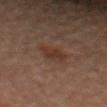workup = imaged on a skin check; not biopsied
subject = male, aged approximately 30
lesion diameter = ~2.5 mm (longest diameter)
TBP lesion metrics = a border-irregularity index near 3/10, internal color variation of about 1.5 on a 0–10 scale, and radial color variation of about 0.5
lighting = cross-polarized
image source = total-body-photography crop, ~15 mm field of view
body site = the head or neck